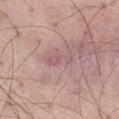{"biopsy_status": "not biopsied; imaged during a skin examination", "image": {"source": "total-body photography crop", "field_of_view_mm": 15}, "site": "leg", "lesion_size": {"long_diameter_mm_approx": 3.5}, "lighting": "white-light", "automated_metrics": {"area_mm2_approx": 3.5, "eccentricity": 0.95, "shape_asymmetry": 0.4, "cielab_L": 57, "cielab_a": 22, "cielab_b": 18, "vs_skin_darker_L": 6.0, "vs_skin_contrast_norm": 5.0, "nevus_likeness_0_100": 0}, "patient": {"sex": "male", "age_approx": 55}}